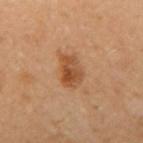Assessment: This lesion was catalogued during total-body skin photography and was not selected for biopsy. Acquisition and patient details: A lesion tile, about 15 mm wide, cut from a 3D total-body photograph. The lesion is on the arm. Automated image analysis of the tile measured an outline eccentricity of about 0.8 (0 = round, 1 = elongated) and a shape-asymmetry score of about 0.25 (0 = symmetric). And it measured a mean CIELAB color near L≈50 a*≈23 b*≈37, roughly 11 lightness units darker than nearby skin, and a lesion-to-skin contrast of about 8 (normalized; higher = more distinct). And it measured border irregularity of about 2.5 on a 0–10 scale, internal color variation of about 5.5 on a 0–10 scale, and peripheral color asymmetry of about 2. A male subject about 65 years old. Approximately 4 mm at its widest.